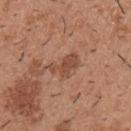Q: Was a biopsy performed?
A: imaged on a skin check; not biopsied
Q: What lighting was used for the tile?
A: white-light illumination
Q: Patient demographics?
A: male, approximately 40 years of age
Q: Lesion location?
A: the upper back
Q: What is the imaging modality?
A: ~15 mm tile from a whole-body skin photo
Q: What did automated image analysis measure?
A: a mean CIELAB color near L≈48 a*≈23 b*≈30, roughly 9 lightness units darker than nearby skin, and a normalized border contrast of about 6.5; border irregularity of about 5.5 on a 0–10 scale, a within-lesion color-variation index near 2/10, and radial color variation of about 0.5